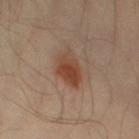Notes:
– biopsy status · total-body-photography surveillance lesion; no biopsy
– tile lighting · cross-polarized
– subject · male, in their 40s
– size · ≈4 mm
– acquisition · total-body-photography crop, ~15 mm field of view
– location · the left thigh
– automated lesion analysis · an area of roughly 10 mm² and an eccentricity of roughly 0.6; a lesion color around L≈44 a*≈21 b*≈29 in CIELAB and a normalized lesion–skin contrast near 8.5; internal color variation of about 5.5 on a 0–10 scale and radial color variation of about 2; a lesion-detection confidence of about 100/100